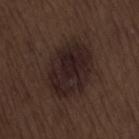A lesion tile, about 15 mm wide, cut from a 3D total-body photograph. Imaged with white-light lighting. The recorded lesion diameter is about 7 mm. The lesion is located on the back. A male patient aged 68–72.